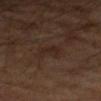Findings:
- biopsy status: catalogued during a skin exam; not biopsied
- size: ≈2.5 mm
- location: the left forearm
- image source: ~15 mm tile from a whole-body skin photo
- automated metrics: a lesion color around L≈23 a*≈14 b*≈21 in CIELAB, roughly 4 lightness units darker than nearby skin, and a normalized lesion–skin contrast near 5.5
- patient: male, aged 63–67
- illumination: cross-polarized illumination